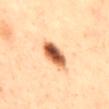Recorded during total-body skin imaging; not selected for excision or biopsy. Longest diameter approximately 4 mm. A male patient aged 48–52. This is a cross-polarized tile. The lesion is located on the mid back. A region of skin cropped from a whole-body photographic capture, roughly 15 mm wide.